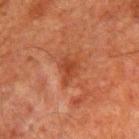Q: Was this lesion biopsied?
A: no biopsy performed (imaged during a skin exam)
Q: Where on the body is the lesion?
A: the left upper arm
Q: What are the patient's age and sex?
A: male, roughly 60 years of age
Q: What did automated image analysis measure?
A: a footprint of about 5.5 mm², a shape eccentricity near 0.65, and a symmetry-axis asymmetry near 0.45; an average lesion color of about L≈35 a*≈25 b*≈30 (CIELAB) and roughly 7 lightness units darker than nearby skin; a border-irregularity index near 4/10, a within-lesion color-variation index near 1.5/10, and radial color variation of about 0.5; a classifier nevus-likeness of about 0/100 and a detector confidence of about 100 out of 100 that the crop contains a lesion
Q: What is the imaging modality?
A: 15 mm crop, total-body photography
Q: What lighting was used for the tile?
A: cross-polarized illumination